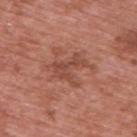Assessment:
Captured during whole-body skin photography for melanoma surveillance; the lesion was not biopsied.